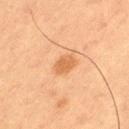The lesion was photographed on a routine skin check and not biopsied; there is no pathology result.
The lesion's longest dimension is about 3 mm.
Located on the leg.
This is a cross-polarized tile.
A 15 mm close-up tile from a total-body photography series done for melanoma screening.
A male subject, in their mid-50s.